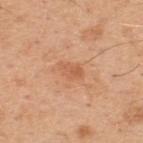| key | value |
|---|---|
| notes | catalogued during a skin exam; not biopsied |
| location | the upper back |
| lesion size | about 3 mm |
| lighting | white-light |
| image-analysis metrics | a footprint of about 4 mm², an outline eccentricity of about 0.8 (0 = round, 1 = elongated), and a symmetry-axis asymmetry near 0.25; border irregularity of about 2.5 on a 0–10 scale and peripheral color asymmetry of about 0.5; an automated nevus-likeness rating near 10 out of 100 and lesion-presence confidence of about 100/100 |
| imaging modality | total-body-photography crop, ~15 mm field of view |
| subject | male, aged 48 to 52 |